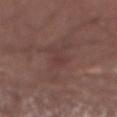<tbp_lesion>
<image>
  <source>total-body photography crop</source>
  <field_of_view_mm>15</field_of_view_mm>
</image>
<patient>
  <sex>male</sex>
  <age_approx>55</age_approx>
</patient>
<lighting>white-light</lighting>
<site>abdomen</site>
</tbp_lesion>